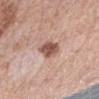Recorded during total-body skin imaging; not selected for excision or biopsy. A female patient aged 63 to 67. The lesion-visualizer software estimated a lesion color around L≈54 a*≈21 b*≈25 in CIELAB. And it measured a border-irregularity rating of about 2.5/10, internal color variation of about 3.5 on a 0–10 scale, and peripheral color asymmetry of about 1. Located on the right forearm. A roughly 15 mm field-of-view crop from a total-body skin photograph. The tile uses white-light illumination.